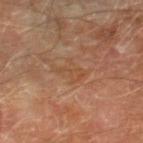Notes:
– workup: total-body-photography surveillance lesion; no biopsy
– imaging modality: 15 mm crop, total-body photography
– location: the right lower leg
– tile lighting: cross-polarized
– size: ≈3.5 mm
– patient: male, about 70 years old